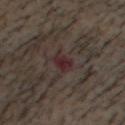<tbp_lesion>
<biopsy_status>not biopsied; imaged during a skin examination</biopsy_status>
<site>head or neck</site>
<automated_metrics>
  <eccentricity>0.8</eccentricity>
  <shape_asymmetry>0.25</shape_asymmetry>
  <cielab_L>26</cielab_L>
  <cielab_a>18</cielab_a>
  <cielab_b>14</cielab_b>
  <vs_skin_contrast_norm>8.5</vs_skin_contrast_norm>
  <border_irregularity_0_10>3.0</border_irregularity_0_10>
  <color_variation_0_10>2.0</color_variation_0_10>
</automated_metrics>
<lighting>cross-polarized</lighting>
<patient>
  <sex>male</sex>
  <age_approx>55</age_approx>
</patient>
<lesion_size>
  <long_diameter_mm_approx>3.0</long_diameter_mm_approx>
</lesion_size>
<image>
  <source>total-body photography crop</source>
  <field_of_view_mm>15</field_of_view_mm>
</image>
</tbp_lesion>